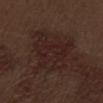Part of a total-body skin-imaging series; this lesion was reviewed on a skin check and was not flagged for biopsy. Cropped from a whole-body photographic skin survey; the tile spans about 15 mm. On the abdomen. A male subject approximately 70 years of age. About 6.5 mm across.